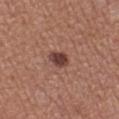workup = total-body-photography surveillance lesion; no biopsy | patient = female, aged approximately 50 | acquisition = ~15 mm tile from a whole-body skin photo | location = the leg | lesion diameter = ~2.5 mm (longest diameter).